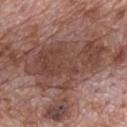{"patient": {"sex": "male", "age_approx": 70}, "image": {"source": "total-body photography crop", "field_of_view_mm": 15}, "automated_metrics": {"eccentricity": 0.85, "shape_asymmetry": 0.45, "vs_skin_darker_L": 10.0, "vs_skin_contrast_norm": 8.0, "border_irregularity_0_10": 8.0, "color_variation_0_10": 4.5, "peripheral_color_asymmetry": 1.5}, "lighting": "white-light", "site": "mid back", "lesion_size": {"long_diameter_mm_approx": 10.5}}